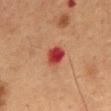Impression: The lesion was tiled from a total-body skin photograph and was not biopsied. Clinical summary: A roughly 15 mm field-of-view crop from a total-body skin photograph. This is a cross-polarized tile. A male subject aged approximately 55. The lesion is on the front of the torso. The lesion's longest dimension is about 3 mm. The total-body-photography lesion software estimated an area of roughly 5.5 mm² and a symmetry-axis asymmetry near 0.2. The analysis additionally found a border-irregularity rating of about 1.5/10, a within-lesion color-variation index near 5.5/10, and peripheral color asymmetry of about 1.5.